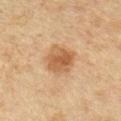<tbp_lesion>
<site>left forearm</site>
<image>
  <source>total-body photography crop</source>
  <field_of_view_mm>15</field_of_view_mm>
</image>
<patient>
  <sex>female</sex>
  <age_approx>45</age_approx>
</patient>
<lesion_size>
  <long_diameter_mm_approx>4.5</long_diameter_mm_approx>
</lesion_size>
</tbp_lesion>